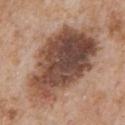About 12 mm across. A male patient aged 63 to 67. The tile uses white-light illumination. On the front of the torso. A 15 mm crop from a total-body photograph taken for skin-cancer surveillance.